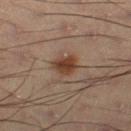The lesion was tiled from a total-body skin photograph and was not biopsied.
Automated image analysis of the tile measured a border-irregularity rating of about 3/10, a color-variation rating of about 2/10, and radial color variation of about 0.5. The software also gave an automated nevus-likeness rating near 90 out of 100 and lesion-presence confidence of about 100/100.
Longest diameter approximately 3 mm.
This is a cross-polarized tile.
The lesion is located on the right thigh.
A 15 mm crop from a total-body photograph taken for skin-cancer surveillance.
A male patient, aged approximately 55.